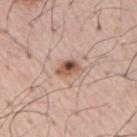Recorded during total-body skin imaging; not selected for excision or biopsy.
Automated image analysis of the tile measured a footprint of about 4 mm² and an eccentricity of roughly 0.75. The analysis additionally found a color-variation rating of about 10/10.
The lesion's longest dimension is about 3 mm.
A roughly 15 mm field-of-view crop from a total-body skin photograph.
A male patient aged around 55.